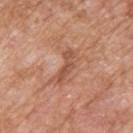No biopsy was performed on this lesion — it was imaged during a full skin examination and was not determined to be concerning. The lesion's longest dimension is about 4 mm. From the upper back. The subject is a male roughly 80 years of age. The tile uses white-light illumination. A 15 mm crop from a total-body photograph taken for skin-cancer surveillance. The total-body-photography lesion software estimated an average lesion color of about L≈52 a*≈24 b*≈32 (CIELAB) and a lesion-to-skin contrast of about 6.5 (normalized; higher = more distinct). It also reported a border-irregularity rating of about 5/10 and a color-variation rating of about 0/10.